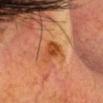Q: Was a biopsy performed?
A: no biopsy performed (imaged during a skin exam)
Q: How was this image acquired?
A: ~15 mm tile from a whole-body skin photo
Q: What are the patient's age and sex?
A: male, in their mid-40s
Q: What is the anatomic site?
A: the head or neck
Q: Lesion size?
A: ~4 mm (longest diameter)